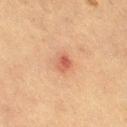Q: Was this lesion biopsied?
A: imaged on a skin check; not biopsied
Q: Lesion location?
A: the right thigh
Q: What kind of image is this?
A: ~15 mm crop, total-body skin-cancer survey
Q: What are the patient's age and sex?
A: female, about 55 years old
Q: How large is the lesion?
A: ≈3 mm